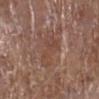The lesion was photographed on a routine skin check and not biopsied; there is no pathology result.
A region of skin cropped from a whole-body photographic capture, roughly 15 mm wide.
A female patient, aged 78–82.
Measured at roughly 5 mm in maximum diameter.
The lesion is located on the left lower leg.
Imaged with white-light lighting.